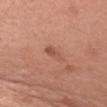Q: Was a biopsy performed?
A: imaged on a skin check; not biopsied
Q: What lighting was used for the tile?
A: white-light
Q: How large is the lesion?
A: about 2.5 mm
Q: Patient demographics?
A: female, aged 48–52
Q: Automated lesion metrics?
A: an area of roughly 3 mm², a shape eccentricity near 0.9, and a shape-asymmetry score of about 0.3 (0 = symmetric); a border-irregularity index near 3/10 and a within-lesion color-variation index near 0.5/10; a nevus-likeness score of about 15/100 and a detector confidence of about 100 out of 100 that the crop contains a lesion
Q: What kind of image is this?
A: ~15 mm crop, total-body skin-cancer survey
Q: Where on the body is the lesion?
A: the left upper arm